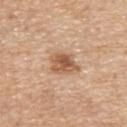notes: total-body-photography surveillance lesion; no biopsy
site: the upper back
lesion size: ~3.5 mm (longest diameter)
image-analysis metrics: a lesion area of about 8 mm² and a shape-asymmetry score of about 0.25 (0 = symmetric); a lesion color around L≈57 a*≈20 b*≈34 in CIELAB, about 12 CIELAB-L* units darker than the surrounding skin, and a lesion-to-skin contrast of about 8 (normalized; higher = more distinct)
tile lighting: white-light illumination
image source: 15 mm crop, total-body photography
subject: male, in their 60s